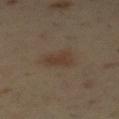Q: Is there a histopathology result?
A: no biopsy performed (imaged during a skin exam)
Q: What is the lesion's diameter?
A: ~3 mm (longest diameter)
Q: How was the tile lit?
A: cross-polarized illumination
Q: Patient demographics?
A: male, approximately 55 years of age
Q: What did automated image analysis measure?
A: border irregularity of about 3.5 on a 0–10 scale; an automated nevus-likeness rating near 95 out of 100 and a detector confidence of about 100 out of 100 that the crop contains a lesion
Q: Lesion location?
A: the mid back
Q: What is the imaging modality?
A: ~15 mm crop, total-body skin-cancer survey